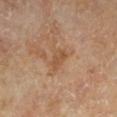Recorded during total-body skin imaging; not selected for excision or biopsy.
A male patient aged around 65.
From the leg.
Cropped from a whole-body photographic skin survey; the tile spans about 15 mm.